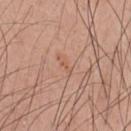Recorded during total-body skin imaging; not selected for excision or biopsy. Located on the chest. The tile uses white-light illumination. A male subject, aged around 50. A region of skin cropped from a whole-body photographic capture, roughly 15 mm wide. Approximately 3 mm at its widest.